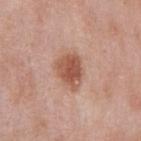| feature | finding |
|---|---|
| workup | no biopsy performed (imaged during a skin exam) |
| image-analysis metrics | a footprint of about 9.5 mm², an outline eccentricity of about 0.65 (0 = round, 1 = elongated), and two-axis asymmetry of about 0.25; a border-irregularity rating of about 2.5/10, a within-lesion color-variation index near 4/10, and radial color variation of about 1.5; an automated nevus-likeness rating near 95 out of 100 and a detector confidence of about 100 out of 100 that the crop contains a lesion |
| patient | male, approximately 55 years of age |
| acquisition | ~15 mm crop, total-body skin-cancer survey |
| anatomic site | the chest |
| lesion size | about 4 mm |
| tile lighting | white-light |